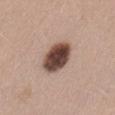No biopsy was performed on this lesion — it was imaged during a full skin examination and was not determined to be concerning. About 4.5 mm across. The tile uses white-light illumination. From the lower back. The lesion-visualizer software estimated an average lesion color of about L≈45 a*≈18 b*≈23 (CIELAB), a lesion–skin lightness drop of about 22, and a normalized lesion–skin contrast near 15. It also reported border irregularity of about 1 on a 0–10 scale, a within-lesion color-variation index near 6/10, and peripheral color asymmetry of about 1.5. A female subject, roughly 40 years of age. Cropped from a total-body skin-imaging series; the visible field is about 15 mm.